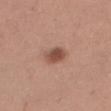Captured during whole-body skin photography for melanoma surveillance; the lesion was not biopsied. Measured at roughly 3 mm in maximum diameter. This image is a 15 mm lesion crop taken from a total-body photograph. Captured under white-light illumination. A female subject aged 28–32. The lesion is located on the right thigh.